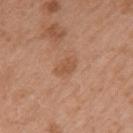The lesion was tiled from a total-body skin photograph and was not biopsied. A female subject, aged around 70. This is a white-light tile. Located on the left upper arm. Measured at roughly 2.5 mm in maximum diameter. Cropped from a whole-body photographic skin survey; the tile spans about 15 mm.